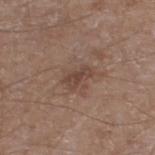No biopsy was performed on this lesion — it was imaged during a full skin examination and was not determined to be concerning.
The lesion is on the leg.
A male subject about 65 years old.
The recorded lesion diameter is about 3 mm.
The tile uses white-light illumination.
A close-up tile cropped from a whole-body skin photograph, about 15 mm across.